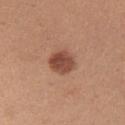Notes:
– biopsy status — no biopsy performed (imaged during a skin exam)
– size — ~3 mm (longest diameter)
– image-analysis metrics — a border-irregularity rating of about 1.5/10, internal color variation of about 3.5 on a 0–10 scale, and peripheral color asymmetry of about 1.5; a nevus-likeness score of about 90/100 and a detector confidence of about 100 out of 100 that the crop contains a lesion
– site — the right upper arm
– acquisition — total-body-photography crop, ~15 mm field of view
– subject — female, approximately 25 years of age